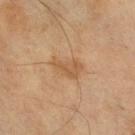Clinical impression:
No biopsy was performed on this lesion — it was imaged during a full skin examination and was not determined to be concerning.
Context:
Located on the right lower leg. A female subject approximately 55 years of age. This is a cross-polarized tile. A close-up tile cropped from a whole-body skin photograph, about 15 mm across.